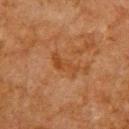{"biopsy_status": "not biopsied; imaged during a skin examination", "image": {"source": "total-body photography crop", "field_of_view_mm": 15}, "lesion_size": {"long_diameter_mm_approx": 4.0}, "automated_metrics": {"area_mm2_approx": 5.0, "shape_asymmetry": 0.45, "border_irregularity_0_10": 5.0, "color_variation_0_10": 1.5, "peripheral_color_asymmetry": 0.0, "nevus_likeness_0_100": 0, "lesion_detection_confidence_0_100": 100}, "site": "upper back", "patient": {"sex": "male", "age_approx": 65}}